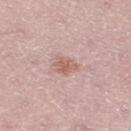No biopsy was performed on this lesion — it was imaged during a full skin examination and was not determined to be concerning.
The lesion is on the right thigh.
The tile uses white-light illumination.
Longest diameter approximately 3 mm.
Automated image analysis of the tile measured an average lesion color of about L≈60 a*≈21 b*≈24 (CIELAB), about 10 CIELAB-L* units darker than the surrounding skin, and a normalized border contrast of about 6.5.
Cropped from a whole-body photographic skin survey; the tile spans about 15 mm.
The patient is a male aged 48–52.